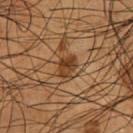follow-up = no biopsy performed (imaged during a skin exam); image source = total-body-photography crop, ~15 mm field of view; lesion diameter = about 3 mm; patient = male, aged 53–57; anatomic site = the chest; lighting = cross-polarized illumination.